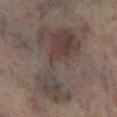Clinical impression:
Imaged during a routine full-body skin examination; the lesion was not biopsied and no histopathology is available.
Clinical summary:
The lesion-visualizer software estimated a lesion color around L≈39 a*≈12 b*≈17 in CIELAB and a normalized border contrast of about 7.5. The analysis additionally found border irregularity of about 9.5 on a 0–10 scale and a peripheral color-asymmetry measure near 2. The software also gave a nevus-likeness score of about 70/100 and a detector confidence of about 90 out of 100 that the crop contains a lesion. The patient is a female aged around 50. Captured under cross-polarized illumination. On the left lower leg. A region of skin cropped from a whole-body photographic capture, roughly 15 mm wide.